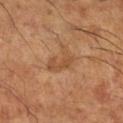Q: Is there a histopathology result?
A: no biopsy performed (imaged during a skin exam)
Q: Where on the body is the lesion?
A: the right lower leg
Q: Who is the patient?
A: male, in their mid- to late 60s
Q: What lighting was used for the tile?
A: cross-polarized
Q: What kind of image is this?
A: 15 mm crop, total-body photography